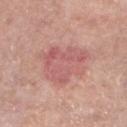follow-up = total-body-photography surveillance lesion; no biopsy | site = the left lower leg | imaging modality = 15 mm crop, total-body photography | lighting = white-light | automated metrics = a classifier nevus-likeness of about 0/100 | patient = male, in their mid- to late 70s | diameter = about 6.5 mm.